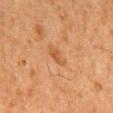follow-up — catalogued during a skin exam; not biopsied | acquisition — total-body-photography crop, ~15 mm field of view | image-analysis metrics — a shape eccentricity near 0.85; border irregularity of about 2.5 on a 0–10 scale, internal color variation of about 2 on a 0–10 scale, and a peripheral color-asymmetry measure near 0.5 | site — the lower back | subject — male, roughly 80 years of age | size — about 2.5 mm.